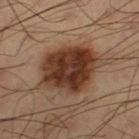Background:
The patient is a male aged around 60. Automated image analysis of the tile measured an area of roughly 30 mm² and a symmetry-axis asymmetry near 0.2. And it measured border irregularity of about 2.5 on a 0–10 scale, a color-variation rating of about 6/10, and radial color variation of about 2. Cropped from a total-body skin-imaging series; the visible field is about 15 mm. Approximately 7.5 mm at its widest. Located on the leg. The tile uses cross-polarized illumination.